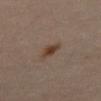Findings:
– biopsy status: catalogued during a skin exam; not biopsied
– anatomic site: the abdomen
– image source: 15 mm crop, total-body photography
– diameter: ≈3 mm
– subject: male, aged 63 to 67